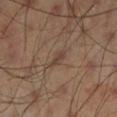Clinical impression: Recorded during total-body skin imaging; not selected for excision or biopsy. Context: Approximately 2.5 mm at its widest. A male subject, in their mid-40s. A 15 mm crop from a total-body photograph taken for skin-cancer surveillance. Automated image analysis of the tile measured a border-irregularity index near 3.5/10 and a color-variation rating of about 0.5/10. The lesion is on the left lower leg.